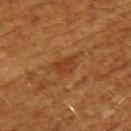biopsy status = total-body-photography surveillance lesion; no biopsy
imaging modality = 15 mm crop, total-body photography
lighting = cross-polarized illumination
patient = male, roughly 60 years of age
site = the back